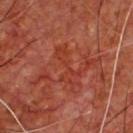follow-up: total-body-photography surveillance lesion; no biopsy | location: the chest | subject: male, aged around 65 | image: total-body-photography crop, ~15 mm field of view.